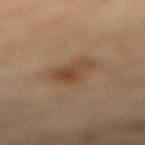follow-up: imaged on a skin check; not biopsied
illumination: cross-polarized
anatomic site: the right lower leg
lesion size: ~4.5 mm (longest diameter)
subject: male, in their mid-80s or older
imaging modality: ~15 mm tile from a whole-body skin photo